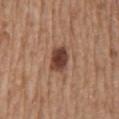workup=catalogued during a skin exam; not biopsied
TBP lesion metrics=a mean CIELAB color near L≈42 a*≈21 b*≈27, about 15 CIELAB-L* units darker than the surrounding skin, and a normalized lesion–skin contrast near 11.5; an automated nevus-likeness rating near 95 out of 100
subject=male, about 70 years old
tile lighting=white-light illumination
lesion diameter=≈3.5 mm
acquisition=~15 mm crop, total-body skin-cancer survey
anatomic site=the mid back